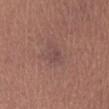Impression: Imaged during a routine full-body skin examination; the lesion was not biopsied and no histopathology is available. Clinical summary: The lesion's longest dimension is about 3.5 mm. This image is a 15 mm lesion crop taken from a total-body photograph. The lesion is located on the right lower leg. Automated tile analysis of the lesion measured a lesion area of about 4.5 mm² and a shape-asymmetry score of about 0.45 (0 = symmetric). And it measured a border-irregularity index near 4.5/10, a color-variation rating of about 1.5/10, and radial color variation of about 0.5. The patient is a female in their mid- to late 60s.